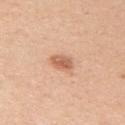{
  "patient": {
    "sex": "female",
    "age_approx": 40
  },
  "image": {
    "source": "total-body photography crop",
    "field_of_view_mm": 15
  },
  "automated_metrics": {
    "area_mm2_approx": 4.5,
    "eccentricity": 0.6,
    "shape_asymmetry": 0.15,
    "nevus_likeness_0_100": 90,
    "lesion_detection_confidence_0_100": 100
  },
  "site": "right upper arm",
  "lighting": "white-light"
}